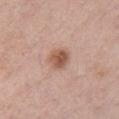| field | value |
|---|---|
| workup | imaged on a skin check; not biopsied |
| lesion size | ≈3 mm |
| patient | female, in their 40s |
| anatomic site | the chest |
| image | total-body-photography crop, ~15 mm field of view |